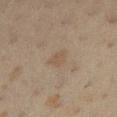Impression:
The lesion was tiled from a total-body skin photograph and was not biopsied.
Acquisition and patient details:
A female subject about 40 years old. The lesion is located on the left lower leg. This is a cross-polarized tile. Cropped from a whole-body photographic skin survey; the tile spans about 15 mm. The total-body-photography lesion software estimated a lesion area of about 4.5 mm², an eccentricity of roughly 0.65, and a shape-asymmetry score of about 0.3 (0 = symmetric). The analysis additionally found about 4 CIELAB-L* units darker than the surrounding skin and a normalized border contrast of about 4.5. It also reported border irregularity of about 3 on a 0–10 scale and a within-lesion color-variation index near 1/10. The analysis additionally found an automated nevus-likeness rating near 0 out of 100 and lesion-presence confidence of about 100/100. The lesion's longest dimension is about 3 mm.